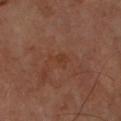This lesion was catalogued during total-body skin photography and was not selected for biopsy. Captured under cross-polarized illumination. A male subject, aged 68 to 72. On the arm. A 15 mm crop from a total-body photograph taken for skin-cancer surveillance.